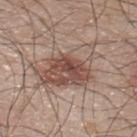This lesion was catalogued during total-body skin photography and was not selected for biopsy. From the upper back. The tile uses white-light illumination. About 7.5 mm across. A male subject, aged 43–47. A close-up tile cropped from a whole-body skin photograph, about 15 mm across.